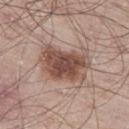Assessment:
Imaged during a routine full-body skin examination; the lesion was not biopsied and no histopathology is available.
Context:
Approximately 6 mm at its widest. On the leg. A 15 mm crop from a total-body photograph taken for skin-cancer surveillance. Imaged with white-light lighting. A male patient, in their mid- to late 60s.